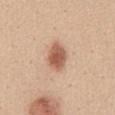No biopsy was performed on this lesion — it was imaged during a full skin examination and was not determined to be concerning.